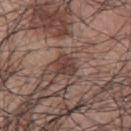Captured during whole-body skin photography for melanoma surveillance; the lesion was not biopsied.
From the back.
Measured at roughly 3 mm in maximum diameter.
This is a white-light tile.
A 15 mm close-up tile from a total-body photography series done for melanoma screening.
The patient is a male approximately 70 years of age.